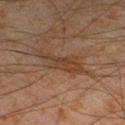biopsy_status: not biopsied; imaged during a skin examination
automated_metrics:
  area_mm2_approx: 8.5
  eccentricity: 0.95
  shape_asymmetry: 0.4
  border_irregularity_0_10: 6.5
  color_variation_0_10: 2.5
  peripheral_color_asymmetry: 1.0
  nevus_likeness_0_100: 0
lighting: cross-polarized
lesion_size:
  long_diameter_mm_approx: 5.5
site: left lower leg
patient:
  sex: male
  age_approx: 45
image:
  source: total-body photography crop
  field_of_view_mm: 15